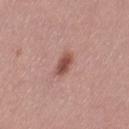Impression: The lesion was tiled from a total-body skin photograph and was not biopsied. Clinical summary: From the left thigh. A lesion tile, about 15 mm wide, cut from a 3D total-body photograph. A female subject about 30 years old.